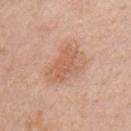Imaged during a routine full-body skin examination; the lesion was not biopsied and no histopathology is available.
An algorithmic analysis of the crop reported a border-irregularity rating of about 3.5/10, internal color variation of about 2.5 on a 0–10 scale, and radial color variation of about 1. The analysis additionally found a classifier nevus-likeness of about 10/100 and a detector confidence of about 100 out of 100 that the crop contains a lesion.
Imaged with white-light lighting.
A roughly 15 mm field-of-view crop from a total-body skin photograph.
Located on the chest.
A male subject roughly 40 years of age.
The recorded lesion diameter is about 5 mm.